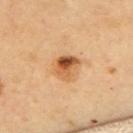| field | value |
|---|---|
| biopsy status | imaged on a skin check; not biopsied |
| image source | 15 mm crop, total-body photography |
| image-analysis metrics | an area of roughly 8 mm², an eccentricity of roughly 0.4, and a symmetry-axis asymmetry near 0.2; a lesion color around L≈59 a*≈24 b*≈41 in CIELAB, roughly 14 lightness units darker than nearby skin, and a normalized lesion–skin contrast near 9 |
| illumination | cross-polarized illumination |
| anatomic site | the upper back |
| patient | female, aged approximately 60 |
| diameter | ~3 mm (longest diameter) |